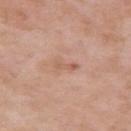Q: Was this lesion biopsied?
A: catalogued during a skin exam; not biopsied
Q: How was this image acquired?
A: total-body-photography crop, ~15 mm field of view
Q: What are the patient's age and sex?
A: male, roughly 55 years of age
Q: Lesion size?
A: ~2.5 mm (longest diameter)
Q: Illumination type?
A: white-light illumination
Q: What is the anatomic site?
A: the right upper arm